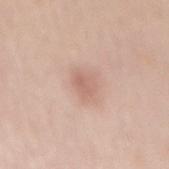follow-up: no biopsy performed (imaged during a skin exam) | size: ~2.5 mm (longest diameter) | tile lighting: white-light illumination | automated lesion analysis: an outline eccentricity of about 0.7 (0 = round, 1 = elongated) and two-axis asymmetry of about 0.25; an average lesion color of about L≈63 a*≈21 b*≈26 (CIELAB) and a normalized lesion–skin contrast near 5.5; a color-variation rating of about 2/10 | patient: female, aged 58–62 | acquisition: ~15 mm tile from a whole-body skin photo | anatomic site: the mid back.